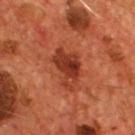Captured during whole-body skin photography for melanoma surveillance; the lesion was not biopsied.
Longest diameter approximately 5 mm.
From the chest.
Captured under cross-polarized illumination.
The patient is a male in their mid- to late 50s.
Automated tile analysis of the lesion measured a lesion area of about 11 mm², an outline eccentricity of about 0.8 (0 = round, 1 = elongated), and two-axis asymmetry of about 0.4. The software also gave a lesion color around L≈35 a*≈30 b*≈33 in CIELAB, about 11 CIELAB-L* units darker than the surrounding skin, and a normalized lesion–skin contrast near 9. It also reported a border-irregularity index near 4.5/10, a within-lesion color-variation index near 5.5/10, and a peripheral color-asymmetry measure near 1.5. It also reported a nevus-likeness score of about 80/100 and lesion-presence confidence of about 100/100.
A 15 mm close-up extracted from a 3D total-body photography capture.